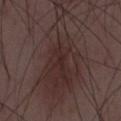- workup: total-body-photography surveillance lesion; no biopsy
- image: ~15 mm crop, total-body skin-cancer survey
- subject: male, aged 48–52
- automated lesion analysis: an average lesion color of about L≈29 a*≈15 b*≈16 (CIELAB) and a normalized lesion–skin contrast near 6.5; a border-irregularity rating of about 7/10 and a color-variation rating of about 4/10; an automated nevus-likeness rating near 75 out of 100 and a detector confidence of about 100 out of 100 that the crop contains a lesion
- lesion diameter: ≈13.5 mm